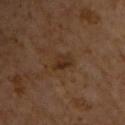biopsy status = catalogued during a skin exam; not biopsied | patient = male, aged approximately 65 | illumination = cross-polarized illumination | anatomic site = the chest | TBP lesion metrics = a within-lesion color-variation index near 3/10; a nevus-likeness score of about 5/100 | size = ~3 mm (longest diameter) | image = ~15 mm tile from a whole-body skin photo.